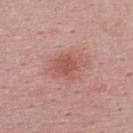Clinical impression: This lesion was catalogued during total-body skin photography and was not selected for biopsy. Clinical summary: A 15 mm close-up tile from a total-body photography series done for melanoma screening. A male patient, in their mid-20s. The lesion's longest dimension is about 4 mm. Located on the upper back.